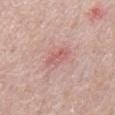Recorded during total-body skin imaging; not selected for excision or biopsy.
Longest diameter approximately 3 mm.
A roughly 15 mm field-of-view crop from a total-body skin photograph.
A male patient in their mid-60s.
Located on the chest.